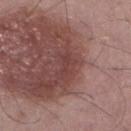Q: Was a biopsy performed?
A: no biopsy performed (imaged during a skin exam)
Q: Illumination type?
A: white-light
Q: What did automated image analysis measure?
A: an outline eccentricity of about 0.8 (0 = round, 1 = elongated) and two-axis asymmetry of about 0.3; border irregularity of about 4.5 on a 0–10 scale, internal color variation of about 0.5 on a 0–10 scale, and a peripheral color-asymmetry measure near 0; an automated nevus-likeness rating near 0 out of 100 and a lesion-detection confidence of about 70/100
Q: Patient demographics?
A: male, approximately 70 years of age
Q: Lesion size?
A: about 3 mm
Q: Lesion location?
A: the left lower leg
Q: How was this image acquired?
A: total-body-photography crop, ~15 mm field of view